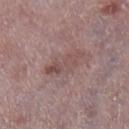* subject: male, in their mid-70s
* site: the left lower leg
* imaging modality: ~15 mm tile from a whole-body skin photo
* lesion diameter: about 5 mm
* tile lighting: white-light
* image-analysis metrics: an eccentricity of roughly 0.95 and a symmetry-axis asymmetry near 0.3